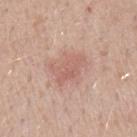follow-up=catalogued during a skin exam; not biopsied | imaging modality=~15 mm tile from a whole-body skin photo | patient=male, in their 30s | anatomic site=the left forearm.